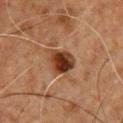This image is a 15 mm lesion crop taken from a total-body photograph.
A male patient, about 60 years old.
The lesion's longest dimension is about 3.5 mm.
From the chest.
The tile uses cross-polarized illumination.